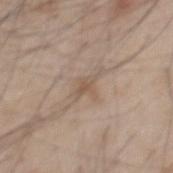Clinical impression:
Recorded during total-body skin imaging; not selected for excision or biopsy.
Clinical summary:
The lesion is located on the mid back. The tile uses white-light illumination. The lesion's longest dimension is about 4 mm. The patient is a male in their mid-40s. A 15 mm crop from a total-body photograph taken for skin-cancer surveillance.